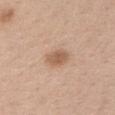<case>
<biopsy_status>not biopsied; imaged during a skin examination</biopsy_status>
<site>head or neck</site>
<patient>
  <sex>female</sex>
  <age_approx>40</age_approx>
</patient>
<lesion_size>
  <long_diameter_mm_approx>3.0</long_diameter_mm_approx>
</lesion_size>
<image>
  <source>total-body photography crop</source>
  <field_of_view_mm>15</field_of_view_mm>
</image>
<lighting>white-light</lighting>
</case>